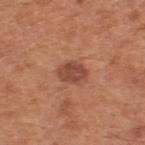Case summary:
– notes — catalogued during a skin exam; not biopsied
– subject — male, aged around 65
– lesion diameter — about 3 mm
– illumination — white-light illumination
– site — the upper back
– acquisition — ~15 mm crop, total-body skin-cancer survey